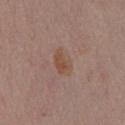workup = catalogued during a skin exam; not biopsied | size = ~3.5 mm (longest diameter) | anatomic site = the chest | acquisition = total-body-photography crop, ~15 mm field of view | tile lighting = white-light | patient = male, aged 53–57.